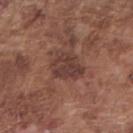Notes:
* follow-up · catalogued during a skin exam; not biopsied
* lesion size · ~4 mm (longest diameter)
* anatomic site · the right forearm
* tile lighting · white-light illumination
* TBP lesion metrics · an area of roughly 11 mm² and a symmetry-axis asymmetry near 0.25; border irregularity of about 2.5 on a 0–10 scale, internal color variation of about 4 on a 0–10 scale, and a peripheral color-asymmetry measure near 1.5
* patient · male, aged 73 to 77
* acquisition · ~15 mm tile from a whole-body skin photo